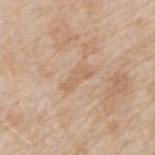Notes:
– biopsy status · no biopsy performed (imaged during a skin exam)
– anatomic site · the right forearm
– illumination · white-light
– patient · male, approximately 45 years of age
– image · ~15 mm crop, total-body skin-cancer survey